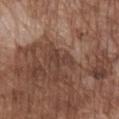Assessment: Part of a total-body skin-imaging series; this lesion was reviewed on a skin check and was not flagged for biopsy. Image and clinical context: From the front of the torso. Cropped from a whole-body photographic skin survey; the tile spans about 15 mm. The patient is a male in their mid- to late 70s.